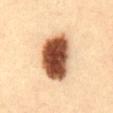Recorded during total-body skin imaging; not selected for excision or biopsy.
The lesion-visualizer software estimated a border-irregularity index near 2/10, a color-variation rating of about 9.5/10, and radial color variation of about 3.
On the abdomen.
Approximately 7 mm at its widest.
Cropped from a whole-body photographic skin survey; the tile spans about 15 mm.
A male patient, aged around 35.
Imaged with cross-polarized lighting.